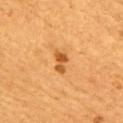- workup: catalogued during a skin exam; not biopsied
- lighting: cross-polarized illumination
- diameter: ~3 mm (longest diameter)
- patient: male, in their mid- to late 50s
- imaging modality: 15 mm crop, total-body photography
- anatomic site: the right upper arm